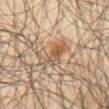biopsy status = no biopsy performed (imaged during a skin exam); subject = male, in their mid-60s; acquisition = 15 mm crop, total-body photography; lighting = cross-polarized illumination; anatomic site = the abdomen.